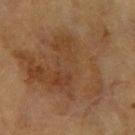Q: Was this lesion biopsied?
A: catalogued during a skin exam; not biopsied
Q: Lesion location?
A: the right forearm
Q: How was this image acquired?
A: ~15 mm crop, total-body skin-cancer survey
Q: Who is the patient?
A: female, aged 58–62